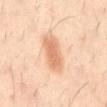| field | value |
|---|---|
| follow-up | imaged on a skin check; not biopsied |
| lesion size | ≈5 mm |
| body site | the mid back |
| automated lesion analysis | an area of roughly 11 mm², an outline eccentricity of about 0.9 (0 = round, 1 = elongated), and two-axis asymmetry of about 0.15; a border-irregularity rating of about 2.5/10, a within-lesion color-variation index near 3/10, and peripheral color asymmetry of about 1 |
| patient | male, aged 38–42 |
| illumination | cross-polarized |
| image | 15 mm crop, total-body photography |